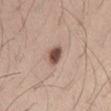No biopsy was performed on this lesion — it was imaged during a full skin examination and was not determined to be concerning. The patient is a male in their mid- to late 20s. This is a white-light tile. A 15 mm close-up tile from a total-body photography series done for melanoma screening. From the lower back.